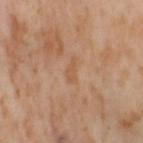| key | value |
|---|---|
| illumination | cross-polarized |
| subject | female, aged 53–57 |
| automated lesion analysis | a classifier nevus-likeness of about 0/100 and lesion-presence confidence of about 100/100 |
| image source | total-body-photography crop, ~15 mm field of view |
| body site | the right thigh |
| lesion size | ≈3 mm |